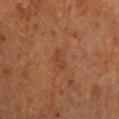  biopsy_status: not biopsied; imaged during a skin examination
  lesion_size:
    long_diameter_mm_approx: 2.5
  patient:
    sex: male
    age_approx: 65
  image:
    source: total-body photography crop
    field_of_view_mm: 15
  lighting: cross-polarized
  site: mid back